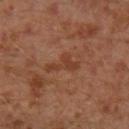Imaged during a routine full-body skin examination; the lesion was not biopsied and no histopathology is available. Captured under cross-polarized illumination. The patient is a male about 30 years old. The lesion is located on the left lower leg. A 15 mm close-up tile from a total-body photography series done for melanoma screening.